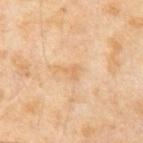Q: Is there a histopathology result?
A: no biopsy performed (imaged during a skin exam)
Q: What did automated image analysis measure?
A: an average lesion color of about L≈67 a*≈19 b*≈39 (CIELAB), a lesion–skin lightness drop of about 6, and a lesion-to-skin contrast of about 5 (normalized; higher = more distinct); border irregularity of about 6 on a 0–10 scale, a within-lesion color-variation index near 0.5/10, and a peripheral color-asymmetry measure near 0.5; an automated nevus-likeness rating near 0 out of 100
Q: Lesion size?
A: ≈2.5 mm
Q: What are the patient's age and sex?
A: male, roughly 65 years of age
Q: How was this image acquired?
A: ~15 mm crop, total-body skin-cancer survey
Q: How was the tile lit?
A: cross-polarized illumination